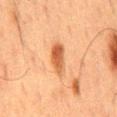Part of a total-body skin-imaging series; this lesion was reviewed on a skin check and was not flagged for biopsy. Automated tile analysis of the lesion measured a footprint of about 7 mm², an outline eccentricity of about 0.9 (0 = round, 1 = elongated), and a symmetry-axis asymmetry near 0.15. The analysis additionally found a color-variation rating of about 5/10 and radial color variation of about 1.5. And it measured an automated nevus-likeness rating near 90 out of 100 and lesion-presence confidence of about 100/100. A male patient about 60 years old. A lesion tile, about 15 mm wide, cut from a 3D total-body photograph. The lesion is on the mid back.